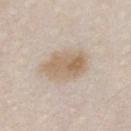Captured during whole-body skin photography for melanoma surveillance; the lesion was not biopsied.
Automated tile analysis of the lesion measured a classifier nevus-likeness of about 45/100.
The lesion is located on the chest.
A male patient in their mid- to late 30s.
A region of skin cropped from a whole-body photographic capture, roughly 15 mm wide.
Imaged with white-light lighting.
The recorded lesion diameter is about 5 mm.